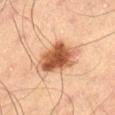Clinical impression: No biopsy was performed on this lesion — it was imaged during a full skin examination and was not determined to be concerning. Clinical summary: A male patient, aged around 70. Cropped from a whole-body photographic skin survey; the tile spans about 15 mm. On the left thigh. The tile uses cross-polarized illumination.